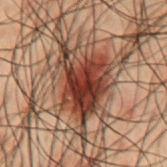Impression: The lesion was tiled from a total-body skin photograph and was not biopsied. Image and clinical context: A 15 mm close-up tile from a total-body photography series done for melanoma screening. A male patient aged 48 to 52. This is a cross-polarized tile. The recorded lesion diameter is about 9.5 mm. The lesion is located on the mid back. Automated tile analysis of the lesion measured a footprint of about 29 mm², a shape eccentricity near 0.8, and two-axis asymmetry of about 0.35. And it measured an average lesion color of about L≈30 a*≈18 b*≈22 (CIELAB), about 13 CIELAB-L* units darker than the surrounding skin, and a normalized border contrast of about 12.